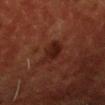- notes — no biopsy performed (imaged during a skin exam)
- image source — 15 mm crop, total-body photography
- patient — male, aged 48–52
- illumination — cross-polarized illumination
- anatomic site — the head or neck
- size — ≈3.5 mm